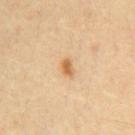biopsy status = no biopsy performed (imaged during a skin exam); automated metrics = a lesion area of about 2.5 mm² and a symmetry-axis asymmetry near 0.35; lighting = cross-polarized; diameter = ~2 mm (longest diameter); subject = male, roughly 60 years of age; anatomic site = the chest; image source = 15 mm crop, total-body photography.